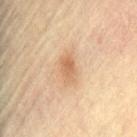Assessment: The lesion was tiled from a total-body skin photograph and was not biopsied. Image and clinical context: This image is a 15 mm lesion crop taken from a total-body photograph. The lesion's longest dimension is about 3.5 mm. A female subject, aged approximately 80. On the lower back.